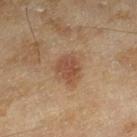Assessment: Captured during whole-body skin photography for melanoma surveillance; the lesion was not biopsied. Background: The tile uses cross-polarized illumination. A 15 mm close-up extracted from a 3D total-body photography capture. A female subject aged 58 to 62. The lesion is located on the right lower leg. Measured at roughly 4 mm in maximum diameter.